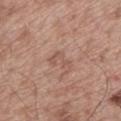The lesion was photographed on a routine skin check and not biopsied; there is no pathology result. A male patient, approximately 55 years of age. On the back. The recorded lesion diameter is about 3.5 mm. A region of skin cropped from a whole-body photographic capture, roughly 15 mm wide. Captured under white-light illumination.